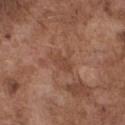Notes:
– follow-up · catalogued during a skin exam; not biopsied
– image · total-body-photography crop, ~15 mm field of view
– site · the chest
– subject · male, aged 73–77